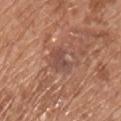Imaged during a routine full-body skin examination; the lesion was not biopsied and no histopathology is available. The lesion is on the chest. A roughly 15 mm field-of-view crop from a total-body skin photograph. The patient is a male in their mid- to late 60s. Longest diameter approximately 2.5 mm. The total-body-photography lesion software estimated a footprint of about 5 mm² and an outline eccentricity of about 0.5 (0 = round, 1 = elongated). The analysis additionally found an average lesion color of about L≈48 a*≈21 b*≈26 (CIELAB), about 7 CIELAB-L* units darker than the surrounding skin, and a normalized border contrast of about 6.